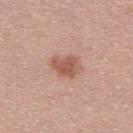This lesion was catalogued during total-body skin photography and was not selected for biopsy. A male patient, approximately 30 years of age. A 15 mm close-up tile from a total-body photography series done for melanoma screening. The recorded lesion diameter is about 3.5 mm. The tile uses white-light illumination.